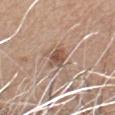Part of a total-body skin-imaging series; this lesion was reviewed on a skin check and was not flagged for biopsy. The subject is a male aged 58–62. The recorded lesion diameter is about 3 mm. Imaged with white-light lighting. The lesion is located on the left upper arm. This image is a 15 mm lesion crop taken from a total-body photograph.